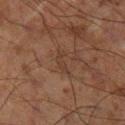| field | value |
|---|---|
| biopsy status | no biopsy performed (imaged during a skin exam) |
| patient | male, approximately 70 years of age |
| acquisition | ~15 mm tile from a whole-body skin photo |
| body site | the right lower leg |